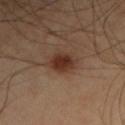Clinical impression:
Imaged during a routine full-body skin examination; the lesion was not biopsied and no histopathology is available.
Acquisition and patient details:
The lesion is on the right upper arm. A region of skin cropped from a whole-body photographic capture, roughly 15 mm wide. A male subject, in their mid-60s.